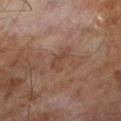<case>
  <image>
    <source>total-body photography crop</source>
    <field_of_view_mm>15</field_of_view_mm>
  </image>
  <patient>
    <sex>male</sex>
    <age_approx>65</age_approx>
  </patient>
</case>